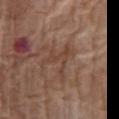The lesion was photographed on a routine skin check and not biopsied; there is no pathology result. Approximately 4.5 mm at its widest. Automated tile analysis of the lesion measured an automated nevus-likeness rating near 0 out of 100 and a lesion-detection confidence of about 50/100. Imaged with white-light lighting. On the abdomen. A lesion tile, about 15 mm wide, cut from a 3D total-body photograph. The subject is a female aged 73–77.